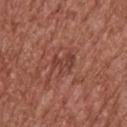| key | value |
|---|---|
| follow-up | catalogued during a skin exam; not biopsied |
| image | ~15 mm tile from a whole-body skin photo |
| lighting | white-light |
| automated lesion analysis | a border-irregularity index near 5/10, internal color variation of about 2.5 on a 0–10 scale, and peripheral color asymmetry of about 1; a detector confidence of about 100 out of 100 that the crop contains a lesion |
| patient | male, in their mid- to late 60s |
| location | the upper back |